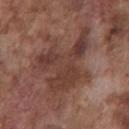workup: total-body-photography surveillance lesion; no biopsy
subject: male, in their mid-70s
automated lesion analysis: a footprint of about 26 mm²; a lesion color around L≈39 a*≈20 b*≈23 in CIELAB; a detector confidence of about 95 out of 100 that the crop contains a lesion
imaging modality: total-body-photography crop, ~15 mm field of view
tile lighting: white-light
anatomic site: the chest
diameter: about 8 mm